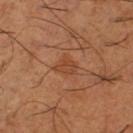Recorded during total-body skin imaging; not selected for excision or biopsy.
Located on the left thigh.
Cropped from a total-body skin-imaging series; the visible field is about 15 mm.
A male subject, about 65 years old.
The tile uses cross-polarized illumination.